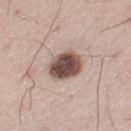The subject is a male approximately 35 years of age.
This image is a 15 mm lesion crop taken from a total-body photograph.
The lesion is on the left thigh.